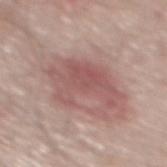| field | value |
|---|---|
| biopsy status | imaged on a skin check; not biopsied |
| image-analysis metrics | a lesion color around L≈54 a*≈22 b*≈22 in CIELAB and a lesion–skin lightness drop of about 9 |
| location | the mid back |
| subject | male, in their 70s |
| diameter | ~6.5 mm (longest diameter) |
| imaging modality | ~15 mm tile from a whole-body skin photo |
| tile lighting | white-light |